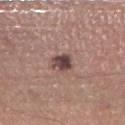automated metrics = border irregularity of about 2 on a 0–10 scale, a color-variation rating of about 4.5/10, and a peripheral color-asymmetry measure near 2; a nevus-likeness score of about 55/100 and lesion-presence confidence of about 100/100
lesion size = about 2.5 mm
tile lighting = white-light illumination
subject = male, in their mid-50s
acquisition = ~15 mm tile from a whole-body skin photo
body site = the right lower leg